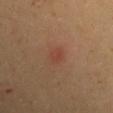The lesion was photographed on a routine skin check and not biopsied; there is no pathology result.
A roughly 15 mm field-of-view crop from a total-body skin photograph.
A male subject aged 53–57.
The lesion's longest dimension is about 2.5 mm.
The lesion is on the left upper arm.